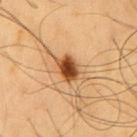| key | value |
|---|---|
| diameter | ~3.5 mm (longest diameter) |
| patient | male, aged around 60 |
| body site | the chest |
| image source | total-body-photography crop, ~15 mm field of view |
| lighting | cross-polarized illumination |